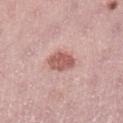{
  "biopsy_status": "not biopsied; imaged during a skin examination",
  "patient": {
    "sex": "female",
    "age_approx": 40
  },
  "automated_metrics": {
    "area_mm2_approx": 6.5,
    "eccentricity": 0.65,
    "shape_asymmetry": 0.15,
    "cielab_L": 57,
    "cielab_a": 25,
    "cielab_b": 24,
    "vs_skin_darker_L": 13.0,
    "vs_skin_contrast_norm": 8.5
  },
  "image": {
    "source": "total-body photography crop",
    "field_of_view_mm": 15
  },
  "lesion_size": {
    "long_diameter_mm_approx": 3.5
  },
  "site": "left lower leg"
}